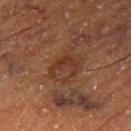The lesion was tiled from a total-body skin photograph and was not biopsied.
The lesion is located on the right thigh.
A roughly 15 mm field-of-view crop from a total-body skin photograph.
A male patient in their mid-70s.
The tile uses cross-polarized illumination.
Approximately 3.5 mm at its widest.